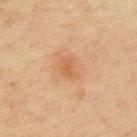Case summary:
* biopsy status — no biopsy performed (imaged during a skin exam)
* lesion size — about 3 mm
* body site — the left upper arm
* image — ~15 mm tile from a whole-body skin photo
* patient — female, aged 53–57